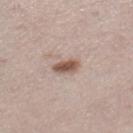Notes:
• location · the leg
• lesion diameter · about 3 mm
• tile lighting · white-light
• imaging modality · ~15 mm crop, total-body skin-cancer survey
• patient · female, aged 38–42
• TBP lesion metrics · a lesion area of about 5 mm², an outline eccentricity of about 0.8 (0 = round, 1 = elongated), and a symmetry-axis asymmetry near 0.3; an average lesion color of about L≈55 a*≈17 b*≈25 (CIELAB), roughly 13 lightness units darker than nearby skin, and a normalized lesion–skin contrast near 9